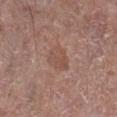Recorded during total-body skin imaging; not selected for excision or biopsy. The patient is a male approximately 65 years of age. A lesion tile, about 15 mm wide, cut from a 3D total-body photograph. From the right lower leg. The tile uses white-light illumination. The lesion's longest dimension is about 3 mm.